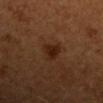This lesion was catalogued during total-body skin photography and was not selected for biopsy. Cropped from a total-body skin-imaging series; the visible field is about 15 mm. The lesion is located on the left upper arm. A male patient approximately 55 years of age. Longest diameter approximately 3 mm. An algorithmic analysis of the crop reported a classifier nevus-likeness of about 85/100 and a detector confidence of about 100 out of 100 that the crop contains a lesion.